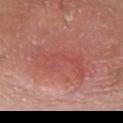follow-up: total-body-photography surveillance lesion; no biopsy | image source: ~15 mm crop, total-body skin-cancer survey | anatomic site: the front of the torso | subject: male, in their 30s | size: about 7 mm.